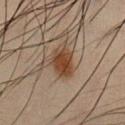Recorded during total-body skin imaging; not selected for excision or biopsy. Located on the chest. A region of skin cropped from a whole-body photographic capture, roughly 15 mm wide. About 3.5 mm across. The tile uses cross-polarized illumination. An algorithmic analysis of the crop reported a lesion area of about 7 mm², an outline eccentricity of about 0.7 (0 = round, 1 = elongated), and a shape-asymmetry score of about 0.25 (0 = symmetric). And it measured a border-irregularity index near 2.5/10, internal color variation of about 3.5 on a 0–10 scale, and radial color variation of about 1. The patient is a male aged 33–37.